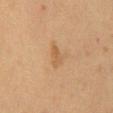biopsy status: catalogued during a skin exam; not biopsied | acquisition: ~15 mm tile from a whole-body skin photo | tile lighting: cross-polarized illumination | body site: the front of the torso | lesion diameter: ≈2.5 mm | patient: female, in their 50s.